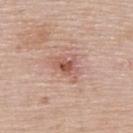The lesion was photographed on a routine skin check and not biopsied; there is no pathology result.
Captured under white-light illumination.
A lesion tile, about 15 mm wide, cut from a 3D total-body photograph.
A female subject, roughly 50 years of age.
Located on the upper back.
Automated tile analysis of the lesion measured a mean CIELAB color near L≈55 a*≈24 b*≈27, a lesion–skin lightness drop of about 11, and a lesion-to-skin contrast of about 7.5 (normalized; higher = more distinct).